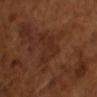No biopsy was performed on this lesion — it was imaged during a full skin examination and was not determined to be concerning.
Imaged with cross-polarized lighting.
Automated tile analysis of the lesion measured an area of roughly 9 mm², an outline eccentricity of about 0.6 (0 = round, 1 = elongated), and two-axis asymmetry of about 0.55.
About 4 mm across.
A male patient about 65 years old.
A region of skin cropped from a whole-body photographic capture, roughly 15 mm wide.